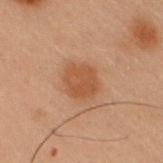Findings:
* workup · no biopsy performed (imaged during a skin exam)
* diameter · about 3.5 mm
* subject · male, approximately 55 years of age
* imaging modality · ~15 mm tile from a whole-body skin photo
* lighting · cross-polarized illumination
* anatomic site · the right upper arm